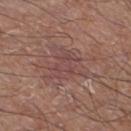notes — total-body-photography surveillance lesion; no biopsy | patient — male, aged 68–72 | tile lighting — white-light illumination | image source — ~15 mm tile from a whole-body skin photo | lesion diameter — about 5 mm | site — the left lower leg.